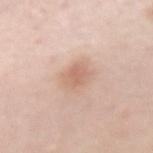Imaged during a routine full-body skin examination; the lesion was not biopsied and no histopathology is available.
A female patient, aged approximately 40.
A 15 mm close-up extracted from a 3D total-body photography capture.
On the right forearm.